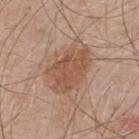Q: Was a biopsy performed?
A: imaged on a skin check; not biopsied
Q: What are the patient's age and sex?
A: male, aged around 50
Q: What lighting was used for the tile?
A: white-light
Q: Where on the body is the lesion?
A: the upper back
Q: What kind of image is this?
A: ~15 mm crop, total-body skin-cancer survey